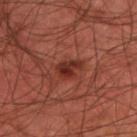* biopsy status — imaged on a skin check; not biopsied
* image — ~15 mm crop, total-body skin-cancer survey
* patient — male, aged approximately 45
* site — the upper back